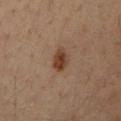The lesion was tiled from a total-body skin photograph and was not biopsied. The lesion is located on the left upper arm. Imaged with cross-polarized lighting. This image is a 15 mm lesion crop taken from a total-body photograph. The lesion-visualizer software estimated an area of roughly 5 mm² and a shape eccentricity near 0.75. The analysis additionally found a lesion color around L≈36 a*≈18 b*≈27 in CIELAB, a lesion–skin lightness drop of about 10, and a normalized border contrast of about 9.5. And it measured a border-irregularity rating of about 2/10, a within-lesion color-variation index near 4/10, and radial color variation of about 1.5. The analysis additionally found a nevus-likeness score of about 95/100 and a detector confidence of about 100 out of 100 that the crop contains a lesion. A male patient approximately 35 years of age.